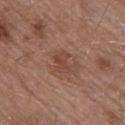Imaged during a routine full-body skin examination; the lesion was not biopsied and no histopathology is available. Captured under white-light illumination. A male patient, roughly 55 years of age. A close-up tile cropped from a whole-body skin photograph, about 15 mm across. The recorded lesion diameter is about 3 mm. The lesion is on the back.